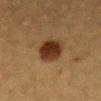workup = total-body-photography surveillance lesion; no biopsy